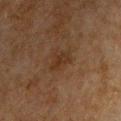biopsy status: no biopsy performed (imaged during a skin exam) | illumination: cross-polarized | image source: ~15 mm tile from a whole-body skin photo | location: the chest | subject: male, roughly 50 years of age.